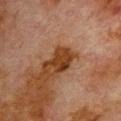Notes:
– follow-up: catalogued during a skin exam; not biopsied
– anatomic site: the upper back
– image-analysis metrics: a border-irregularity index near 4.5/10 and a color-variation rating of about 3/10; a nevus-likeness score of about 10/100
– lighting: cross-polarized
– patient: male, aged around 80
– imaging modality: total-body-photography crop, ~15 mm field of view
– lesion size: ~3.5 mm (longest diameter)